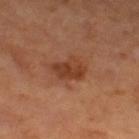Imaged during a routine full-body skin examination; the lesion was not biopsied and no histopathology is available. A close-up tile cropped from a whole-body skin photograph, about 15 mm across. Located on the right thigh. Captured under cross-polarized illumination. Automated image analysis of the tile measured an area of roughly 8 mm², an eccentricity of roughly 0.7, and two-axis asymmetry of about 0.3. And it measured an automated nevus-likeness rating near 45 out of 100 and lesion-presence confidence of about 100/100. A female subject, about 65 years old.